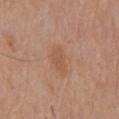Context: A 15 mm crop from a total-body photograph taken for skin-cancer surveillance. The patient is a male aged around 80. The lesion is on the chest.